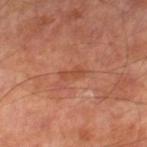Recorded during total-body skin imaging; not selected for excision or biopsy. A male patient about 70 years old. Captured under cross-polarized illumination. A lesion tile, about 15 mm wide, cut from a 3D total-body photograph. On the right lower leg.